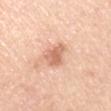Q: Was a biopsy performed?
A: catalogued during a skin exam; not biopsied
Q: Patient demographics?
A: male, about 50 years old
Q: Lesion location?
A: the left upper arm
Q: What kind of image is this?
A: 15 mm crop, total-body photography
Q: What lighting was used for the tile?
A: white-light illumination